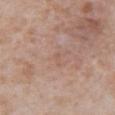{
  "biopsy_status": "not biopsied; imaged during a skin examination",
  "site": "abdomen",
  "image": {
    "source": "total-body photography crop",
    "field_of_view_mm": 15
  },
  "patient": {
    "sex": "male",
    "age_approx": 65
  }
}